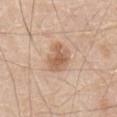workup: catalogued during a skin exam; not biopsied
tile lighting: white-light
lesion diameter: ~3.5 mm (longest diameter)
site: the mid back
image-analysis metrics: a lesion area of about 7 mm², an eccentricity of roughly 0.75, and two-axis asymmetry of about 0.3; a lesion color around L≈60 a*≈20 b*≈31 in CIELAB and a lesion–skin lightness drop of about 11; a color-variation rating of about 2.5/10 and a peripheral color-asymmetry measure near 0.5
subject: male, approximately 80 years of age
image source: ~15 mm crop, total-body skin-cancer survey